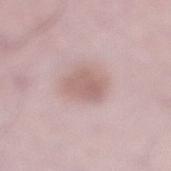notes = total-body-photography surveillance lesion; no biopsy
diameter = ≈3.5 mm
location = the right lower leg
image source = ~15 mm tile from a whole-body skin photo
tile lighting = white-light
patient = male, aged 48 to 52